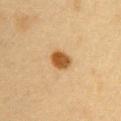| key | value |
|---|---|
| follow-up | total-body-photography surveillance lesion; no biopsy |
| patient | female, roughly 30 years of age |
| body site | the right upper arm |
| imaging modality | 15 mm crop, total-body photography |
| lighting | cross-polarized |
| automated metrics | a shape eccentricity near 0.6; about 14 CIELAB-L* units darker than the surrounding skin and a normalized lesion–skin contrast near 11; a classifier nevus-likeness of about 100/100 and lesion-presence confidence of about 100/100 |
| size | about 3 mm |